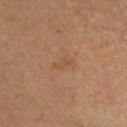| key | value |
|---|---|
| workup | total-body-photography surveillance lesion; no biopsy |
| image-analysis metrics | an area of roughly 3 mm² and an eccentricity of roughly 0.85; an average lesion color of about L≈40 a*≈16 b*≈29 (CIELAB), roughly 4 lightness units darker than nearby skin, and a normalized lesion–skin contrast near 4.5; a classifier nevus-likeness of about 0/100 and lesion-presence confidence of about 100/100 |
| image | ~15 mm crop, total-body skin-cancer survey |
| location | the front of the torso |
| lighting | cross-polarized |
| patient | male, aged approximately 40 |
| size | ≈2.5 mm |